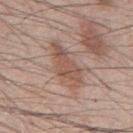Captured during whole-body skin photography for melanoma surveillance; the lesion was not biopsied. A roughly 15 mm field-of-view crop from a total-body skin photograph. On the mid back. The subject is a male about 45 years old. About 5.5 mm across. Captured under white-light illumination.